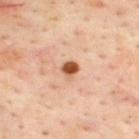The lesion was tiled from a total-body skin photograph and was not biopsied. Automated tile analysis of the lesion measured an area of roughly 3.5 mm², an eccentricity of roughly 0.45, and two-axis asymmetry of about 0.25. And it measured a lesion-detection confidence of about 100/100. Imaged with cross-polarized lighting. The lesion is on the upper back. A lesion tile, about 15 mm wide, cut from a 3D total-body photograph. A male subject roughly 45 years of age. The lesion's longest dimension is about 2 mm.